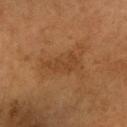Part of a total-body skin-imaging series; this lesion was reviewed on a skin check and was not flagged for biopsy.